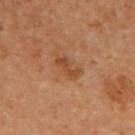This lesion was catalogued during total-body skin photography and was not selected for biopsy.
The subject is a female approximately 55 years of age.
The lesion's longest dimension is about 3 mm.
The lesion is on the upper back.
This is a cross-polarized tile.
Automated image analysis of the tile measured an average lesion color of about L≈44 a*≈23 b*≈34 (CIELAB), a lesion–skin lightness drop of about 8, and a normalized lesion–skin contrast near 6.5. The analysis additionally found a border-irregularity index near 4/10. And it measured a classifier nevus-likeness of about 5/100 and a detector confidence of about 100 out of 100 that the crop contains a lesion.
A 15 mm close-up tile from a total-body photography series done for melanoma screening.